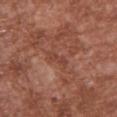Assessment:
The lesion was tiled from a total-body skin photograph and was not biopsied.
Acquisition and patient details:
The lesion's longest dimension is about 3 mm. A region of skin cropped from a whole-body photographic capture, roughly 15 mm wide. Captured under white-light illumination. The subject is a male approximately 45 years of age. The lesion is on the chest.